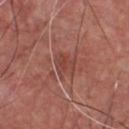<case>
<biopsy_status>not biopsied; imaged during a skin examination</biopsy_status>
<image>
  <source>total-body photography crop</source>
  <field_of_view_mm>15</field_of_view_mm>
</image>
<site>chest</site>
<patient>
  <sex>male</sex>
  <age_approx>65</age_approx>
</patient>
<automated_metrics>
  <nevus_likeness_0_100>0</nevus_likeness_0_100>
  <lesion_detection_confidence_0_100>90</lesion_detection_confidence_0_100>
</automated_metrics>
</case>